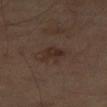The lesion was tiled from a total-body skin photograph and was not biopsied.
A 15 mm crop from a total-body photograph taken for skin-cancer surveillance.
Longest diameter approximately 3 mm.
On the left leg.
Captured under cross-polarized illumination.
The total-body-photography lesion software estimated a lesion color around L≈26 a*≈14 b*≈20 in CIELAB, roughly 6 lightness units darker than nearby skin, and a normalized lesion–skin contrast near 7. The software also gave a classifier nevus-likeness of about 15/100 and lesion-presence confidence of about 100/100.
A male patient in their mid-60s.